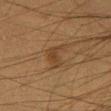notes = no biopsy performed (imaged during a skin exam)
image = total-body-photography crop, ~15 mm field of view
lesion diameter = ~4 mm (longest diameter)
anatomic site = the front of the torso
subject = male, aged 48 to 52
illumination = cross-polarized